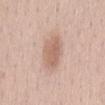biopsy_status: not biopsied; imaged during a skin examination
patient:
  sex: female
  age_approx: 60
site: lower back
automated_metrics:
  area_mm2_approx: 11.0
  eccentricity: 0.6
  shape_asymmetry: 0.15
  cielab_L: 63
  cielab_a: 19
  cielab_b: 27
  vs_skin_darker_L: 10.0
  vs_skin_contrast_norm: 6.5
  peripheral_color_asymmetry: 1.0
  nevus_likeness_0_100: 60
  lesion_detection_confidence_0_100: 100
lighting: white-light
image:
  source: total-body photography crop
  field_of_view_mm: 15
lesion_size:
  long_diameter_mm_approx: 4.0